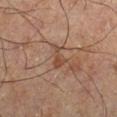Q: Is there a histopathology result?
A: total-body-photography surveillance lesion; no biopsy
Q: Lesion location?
A: the right lower leg
Q: What lighting was used for the tile?
A: cross-polarized
Q: Automated lesion metrics?
A: a lesion area of about 3.5 mm² and a shape-asymmetry score of about 0.45 (0 = symmetric); roughly 7 lightness units darker than nearby skin and a lesion-to-skin contrast of about 6.5 (normalized; higher = more distinct); an automated nevus-likeness rating near 0 out of 100 and a lesion-detection confidence of about 100/100
Q: What is the imaging modality?
A: 15 mm crop, total-body photography
Q: Who is the patient?
A: male, in their mid- to late 60s